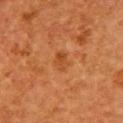This lesion was catalogued during total-body skin photography and was not selected for biopsy. A roughly 15 mm field-of-view crop from a total-body skin photograph. From the left upper arm. This is a cross-polarized tile. A female patient, about 50 years old.